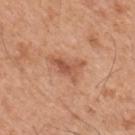Impression: Part of a total-body skin-imaging series; this lesion was reviewed on a skin check and was not flagged for biopsy. Image and clinical context: The subject is a male aged 43–47. This is a white-light tile. The lesion's longest dimension is about 4 mm. A close-up tile cropped from a whole-body skin photograph, about 15 mm across. Automated tile analysis of the lesion measured a border-irregularity rating of about 6.5/10 and radial color variation of about 1. The analysis additionally found a detector confidence of about 100 out of 100 that the crop contains a lesion. The lesion is located on the upper back.